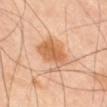biopsy_status: not biopsied; imaged during a skin examination
patient:
  sex: male
  age_approx: 65
image:
  source: total-body photography crop
  field_of_view_mm: 15
site: leg
lesion_size:
  long_diameter_mm_approx: 5.0